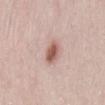Background: A female subject roughly 30 years of age. From the abdomen. A 15 mm close-up tile from a total-body photography series done for melanoma screening. Longest diameter approximately 3 mm.